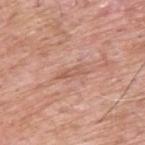| field | value |
|---|---|
| notes | catalogued during a skin exam; not biopsied |
| acquisition | total-body-photography crop, ~15 mm field of view |
| anatomic site | the back |
| illumination | white-light |
| patient | male, about 60 years old |
| lesion diameter | ~3 mm (longest diameter) |